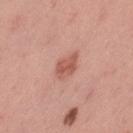Part of a total-body skin-imaging series; this lesion was reviewed on a skin check and was not flagged for biopsy. Cropped from a whole-body photographic skin survey; the tile spans about 15 mm. From the left thigh. The lesion-visualizer software estimated a lesion area of about 5.5 mm², a shape eccentricity near 0.8, and a symmetry-axis asymmetry near 0.25. The software also gave border irregularity of about 2 on a 0–10 scale, a color-variation rating of about 2.5/10, and peripheral color asymmetry of about 1. A female patient about 35 years old.